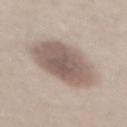The lesion was photographed on a routine skin check and not biopsied; there is no pathology result. The lesion's longest dimension is about 7 mm. The tile uses white-light illumination. From the back. The patient is a male roughly 30 years of age. Automated tile analysis of the lesion measured a nevus-likeness score of about 50/100 and a lesion-detection confidence of about 25/100. Cropped from a whole-body photographic skin survey; the tile spans about 15 mm.